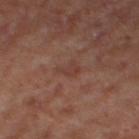lesion diameter — about 3 mm
subject — female
location — the leg
image-analysis metrics — an average lesion color of about L≈40 a*≈21 b*≈25 (CIELAB), roughly 5 lightness units darker than nearby skin, and a normalized border contrast of about 4.5; border irregularity of about 4.5 on a 0–10 scale and a color-variation rating of about 1/10; lesion-presence confidence of about 100/100
image — ~15 mm crop, total-body skin-cancer survey